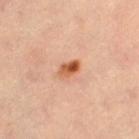{
  "biopsy_status": "not biopsied; imaged during a skin examination",
  "image": {
    "source": "total-body photography crop",
    "field_of_view_mm": 15
  },
  "patient": {
    "sex": "female",
    "age_approx": 35
  },
  "site": "left thigh",
  "lesion_size": {
    "long_diameter_mm_approx": 2.5
  },
  "lighting": "cross-polarized",
  "automated_metrics": {
    "cielab_L": 57,
    "cielab_a": 26,
    "cielab_b": 37,
    "vs_skin_darker_L": 13.0,
    "vs_skin_contrast_norm": 9.5
  }
}